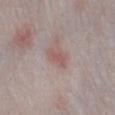Notes:
– workup: catalogued during a skin exam; not biopsied
– image: 15 mm crop, total-body photography
– subject: male, approximately 65 years of age
– location: the left lower leg
– image-analysis metrics: a border-irregularity index near 3.5/10, a color-variation rating of about 2/10, and peripheral color asymmetry of about 1
– tile lighting: white-light
– lesion diameter: ~3 mm (longest diameter)